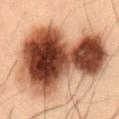The lesion was tiled from a total-body skin photograph and was not biopsied.
Located on the abdomen.
A region of skin cropped from a whole-body photographic capture, roughly 15 mm wide.
The subject is a male approximately 55 years of age.